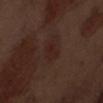<tbp_lesion>
<biopsy_status>not biopsied; imaged during a skin examination</biopsy_status>
<site>abdomen</site>
<lighting>white-light</lighting>
<patient>
  <sex>male</sex>
  <age_approx>70</age_approx>
</patient>
<image>
  <source>total-body photography crop</source>
  <field_of_view_mm>15</field_of_view_mm>
</image>
<lesion_size>
  <long_diameter_mm_approx>2.5</long_diameter_mm_approx>
</lesion_size>
<automated_metrics>
  <eccentricity>0.8</eccentricity>
  <shape_asymmetry>0.35</shape_asymmetry>
  <nevus_likeness_0_100>40</nevus_likeness_0_100>
  <lesion_detection_confidence_0_100>100</lesion_detection_confidence_0_100>
</automated_metrics>
</tbp_lesion>